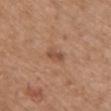  biopsy_status: not biopsied; imaged during a skin examination
  site: chest
  patient:
    sex: female
    age_approx: 70
  lesion_size:
    long_diameter_mm_approx: 2.5
  lighting: white-light
  image:
    source: total-body photography crop
    field_of_view_mm: 15
  automated_metrics:
    area_mm2_approx: 3.5
    eccentricity: 0.8
    shape_asymmetry: 0.3
    cielab_L: 50
    cielab_a: 22
    cielab_b: 31
    vs_skin_darker_L: 9.0
    vs_skin_contrast_norm: 6.5